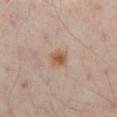- biopsy status — catalogued during a skin exam; not biopsied
- automated lesion analysis — a mean CIELAB color near L≈55 a*≈18 b*≈30, roughly 9 lightness units darker than nearby skin, and a lesion-to-skin contrast of about 8 (normalized; higher = more distinct); an automated nevus-likeness rating near 95 out of 100 and lesion-presence confidence of about 100/100
- tile lighting — cross-polarized
- anatomic site — the abdomen
- image source — total-body-photography crop, ~15 mm field of view
- subject — male, aged around 50
- diameter — ≈2.5 mm